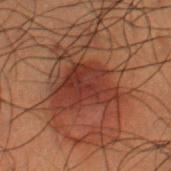This lesion was catalogued during total-body skin photography and was not selected for biopsy. Measured at roughly 5.5 mm in maximum diameter. A male patient, aged approximately 50. The lesion is located on the right lower leg. A 15 mm crop from a total-body photograph taken for skin-cancer surveillance. Captured under cross-polarized illumination.